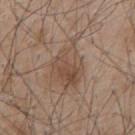patient — male, in their mid- to late 40s
tile lighting — white-light
lesion diameter — ≈6 mm
acquisition — total-body-photography crop, ~15 mm field of view
body site — the mid back
image-analysis metrics — a footprint of about 13 mm², an eccentricity of roughly 0.75, and a shape-asymmetry score of about 0.3 (0 = symmetric); an average lesion color of about L≈48 a*≈16 b*≈27 (CIELAB), a lesion–skin lightness drop of about 8, and a lesion-to-skin contrast of about 6.5 (normalized; higher = more distinct); internal color variation of about 5.5 on a 0–10 scale